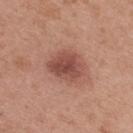| feature | finding |
|---|---|
| workup | no biopsy performed (imaged during a skin exam) |
| anatomic site | the upper back |
| image source | ~15 mm crop, total-body skin-cancer survey |
| automated lesion analysis | a border-irregularity index near 2/10, internal color variation of about 4 on a 0–10 scale, and peripheral color asymmetry of about 1.5; a nevus-likeness score of about 85/100 |
| size | ~4.5 mm (longest diameter) |
| patient | female, about 40 years old |